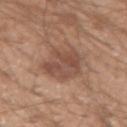{
  "biopsy_status": "not biopsied; imaged during a skin examination",
  "patient": {
    "sex": "male",
    "age_approx": 20
  },
  "image": {
    "source": "total-body photography crop",
    "field_of_view_mm": 15
  },
  "site": "right upper arm"
}